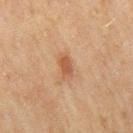follow-up: imaged on a skin check; not biopsied
anatomic site: the leg
tile lighting: cross-polarized
subject: female, in their 60s
diameter: ~2.5 mm (longest diameter)
image-analysis metrics: a mean CIELAB color near L≈51 a*≈21 b*≈33, roughly 9 lightness units darker than nearby skin, and a normalized border contrast of about 7; a border-irregularity rating of about 3/10, a color-variation rating of about 2/10, and a peripheral color-asymmetry measure near 0.5
image source: ~15 mm tile from a whole-body skin photo